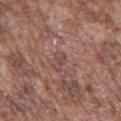This lesion was catalogued during total-body skin photography and was not selected for biopsy. Imaged with white-light lighting. A male subject, in their mid- to late 70s. From the chest. The total-body-photography lesion software estimated an area of roughly 2.5 mm², a shape eccentricity near 0.85, and two-axis asymmetry of about 0.55. It also reported an average lesion color of about L≈47 a*≈19 b*≈22 (CIELAB), roughly 7 lightness units darker than nearby skin, and a normalized border contrast of about 5.5. A lesion tile, about 15 mm wide, cut from a 3D total-body photograph. The recorded lesion diameter is about 2.5 mm.